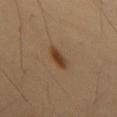Clinical impression: The lesion was tiled from a total-body skin photograph and was not biopsied. Clinical summary: Measured at roughly 3.5 mm in maximum diameter. A 15 mm close-up tile from a total-body photography series done for melanoma screening. A male patient, roughly 55 years of age. The lesion is on the chest.